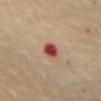{
  "biopsy_status": "not biopsied; imaged during a skin examination",
  "site": "front of the torso",
  "patient": {
    "sex": "female",
    "age_approx": 60
  },
  "automated_metrics": {
    "area_mm2_approx": 4.0,
    "eccentricity": 0.55,
    "shape_asymmetry": 0.15
  },
  "image": {
    "source": "total-body photography crop",
    "field_of_view_mm": 15
  },
  "lesion_size": {
    "long_diameter_mm_approx": 2.5
  },
  "lighting": "cross-polarized"
}